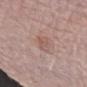Acquisition and patient details:
This is a white-light tile. A lesion tile, about 15 mm wide, cut from a 3D total-body photograph. From the arm. The patient is a female in their mid-60s.